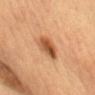Assessment: Captured during whole-body skin photography for melanoma surveillance; the lesion was not biopsied. Background: The recorded lesion diameter is about 4 mm. A female subject, approximately 60 years of age. On the chest. Automated tile analysis of the lesion measured an average lesion color of about L≈48 a*≈22 b*≈34 (CIELAB), roughly 12 lightness units darker than nearby skin, and a lesion-to-skin contrast of about 9 (normalized; higher = more distinct). The analysis additionally found a border-irregularity rating of about 2.5/10 and a within-lesion color-variation index near 7/10. Cropped from a total-body skin-imaging series; the visible field is about 15 mm. Captured under cross-polarized illumination.